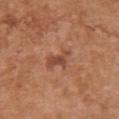Captured during whole-body skin photography for melanoma surveillance; the lesion was not biopsied.
This image is a 15 mm lesion crop taken from a total-body photograph.
Measured at roughly 4 mm in maximum diameter.
Captured under white-light illumination.
A female subject approximately 65 years of age.
Automated image analysis of the tile measured a lesion color around L≈49 a*≈24 b*≈32 in CIELAB, about 7 CIELAB-L* units darker than the surrounding skin, and a lesion-to-skin contrast of about 5.5 (normalized; higher = more distinct). The analysis additionally found a border-irregularity index near 5/10 and a within-lesion color-variation index near 3.5/10. And it measured a classifier nevus-likeness of about 0/100 and lesion-presence confidence of about 100/100.
The lesion is located on the chest.